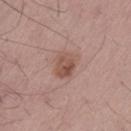Assessment: Part of a total-body skin-imaging series; this lesion was reviewed on a skin check and was not flagged for biopsy. Background: A male subject, roughly 55 years of age. The tile uses white-light illumination. A close-up tile cropped from a whole-body skin photograph, about 15 mm across. Automated tile analysis of the lesion measured an area of roughly 7.5 mm² and a shape-asymmetry score of about 0.2 (0 = symmetric). The software also gave a mean CIELAB color near L≈52 a*≈20 b*≈25, roughly 9 lightness units darker than nearby skin, and a normalized border contrast of about 7. And it measured border irregularity of about 2 on a 0–10 scale and peripheral color asymmetry of about 2. It also reported a nevus-likeness score of about 25/100 and a detector confidence of about 100 out of 100 that the crop contains a lesion.